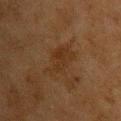| field | value |
|---|---|
| biopsy status | catalogued during a skin exam; not biopsied |
| body site | the upper back |
| size | ≈4.5 mm |
| imaging modality | ~15 mm crop, total-body skin-cancer survey |
| patient | male, aged 73–77 |
| illumination | cross-polarized illumination |
| image-analysis metrics | a lesion area of about 8.5 mm², an eccentricity of roughly 0.85, and a shape-asymmetry score of about 0.3 (0 = symmetric); border irregularity of about 4 on a 0–10 scale, internal color variation of about 2.5 on a 0–10 scale, and radial color variation of about 1; a nevus-likeness score of about 5/100 and lesion-presence confidence of about 100/100 |